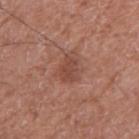Q: Was a biopsy performed?
A: no biopsy performed (imaged during a skin exam)
Q: Automated lesion metrics?
A: a mean CIELAB color near L≈46 a*≈23 b*≈27 and roughly 8 lightness units darker than nearby skin; a detector confidence of about 100 out of 100 that the crop contains a lesion
Q: What is the lesion's diameter?
A: about 2.5 mm
Q: How was this image acquired?
A: ~15 mm crop, total-body skin-cancer survey
Q: Patient demographics?
A: male, aged approximately 75
Q: What is the anatomic site?
A: the right upper arm
Q: How was the tile lit?
A: white-light